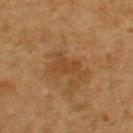Q: Was this lesion biopsied?
A: imaged on a skin check; not biopsied
Q: What is the imaging modality?
A: 15 mm crop, total-body photography
Q: What is the anatomic site?
A: the upper back
Q: Patient demographics?
A: male, in their mid-70s